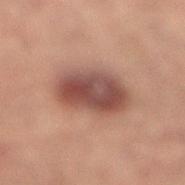Assessment:
This lesion was catalogued during total-body skin photography and was not selected for biopsy.
Background:
On the right lower leg. Longest diameter approximately 6 mm. Captured under cross-polarized illumination. A 15 mm close-up tile from a total-body photography series done for melanoma screening. The subject is a female roughly 70 years of age. Automated image analysis of the tile measured a lesion area of about 20 mm², an outline eccentricity of about 0.7 (0 = round, 1 = elongated), and two-axis asymmetry of about 0.1. And it measured a lesion color around L≈40 a*≈19 b*≈21 in CIELAB and a lesion-to-skin contrast of about 10 (normalized; higher = more distinct). It also reported a nevus-likeness score of about 95/100 and lesion-presence confidence of about 100/100.